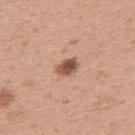Findings:
* notes · catalogued during a skin exam; not biopsied
* illumination · white-light illumination
* lesion diameter · ~2.5 mm (longest diameter)
* TBP lesion metrics · a lesion color around L≈53 a*≈22 b*≈30 in CIELAB, about 15 CIELAB-L* units darker than the surrounding skin, and a lesion-to-skin contrast of about 10 (normalized; higher = more distinct); a border-irregularity index near 2/10 and a peripheral color-asymmetry measure near 1.5; a nevus-likeness score of about 95/100
* patient · male, approximately 30 years of age
* imaging modality · ~15 mm crop, total-body skin-cancer survey
* anatomic site · the upper back